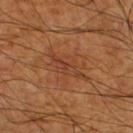notes = catalogued during a skin exam; not biopsied | location = the right lower leg | patient = male, approximately 60 years of age | imaging modality = ~15 mm tile from a whole-body skin photo | lesion diameter = ~5 mm (longest diameter).